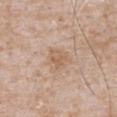{"image": {"source": "total-body photography crop", "field_of_view_mm": 15}, "site": "abdomen", "lesion_size": {"long_diameter_mm_approx": 2.5}, "lighting": "white-light", "patient": {"sex": "male", "age_approx": 65}, "automated_metrics": {"cielab_L": 60, "cielab_a": 18, "cielab_b": 31, "vs_skin_darker_L": 7.0, "border_irregularity_0_10": 2.5, "color_variation_0_10": 3.0, "peripheral_color_asymmetry": 1.0, "nevus_likeness_0_100": 0, "lesion_detection_confidence_0_100": 100}}